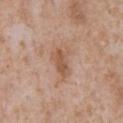{
  "biopsy_status": "not biopsied; imaged during a skin examination",
  "lesion_size": {
    "long_diameter_mm_approx": 4.0
  },
  "site": "chest",
  "image": {
    "source": "total-body photography crop",
    "field_of_view_mm": 15
  },
  "lighting": "white-light",
  "patient": {
    "sex": "male",
    "age_approx": 65
  }
}